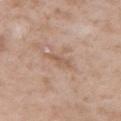biopsy status: imaged on a skin check; not biopsied | anatomic site: the right upper arm | lesion size: ~3 mm (longest diameter) | subject: male, aged approximately 50 | imaging modality: total-body-photography crop, ~15 mm field of view | TBP lesion metrics: a lesion area of about 3 mm², an outline eccentricity of about 0.9 (0 = round, 1 = elongated), and two-axis asymmetry of about 0.35; internal color variation of about 0 on a 0–10 scale; a classifier nevus-likeness of about 0/100 and lesion-presence confidence of about 100/100 | illumination: white-light.